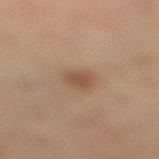notes: total-body-photography surveillance lesion; no biopsy | body site: the leg | imaging modality: total-body-photography crop, ~15 mm field of view | illumination: cross-polarized illumination | patient: female, aged 68–72.